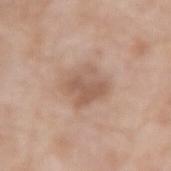Imaged during a routine full-body skin examination; the lesion was not biopsied and no histopathology is available.
Approximately 4.5 mm at its widest.
The tile uses white-light illumination.
The lesion is on the right forearm.
A region of skin cropped from a whole-body photographic capture, roughly 15 mm wide.
The patient is a female roughly 75 years of age.